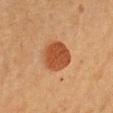The lesion was tiled from a total-body skin photograph and was not biopsied. A female patient, aged around 40. Imaged with cross-polarized lighting. Cropped from a whole-body photographic skin survey; the tile spans about 15 mm. On the front of the torso.